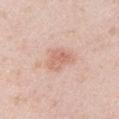Clinical impression:
The lesion was photographed on a routine skin check and not biopsied; there is no pathology result.
Context:
The lesion is on the left upper arm. A 15 mm close-up tile from a total-body photography series done for melanoma screening. Imaged with white-light lighting. The lesion's longest dimension is about 3.5 mm. A male subject, approximately 40 years of age. The lesion-visualizer software estimated a lesion area of about 8 mm², an eccentricity of roughly 0.55, and two-axis asymmetry of about 0.3. The analysis additionally found border irregularity of about 3 on a 0–10 scale, a color-variation rating of about 3/10, and radial color variation of about 1.